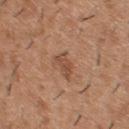Findings:
- notes: total-body-photography surveillance lesion; no biopsy